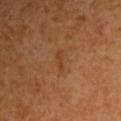Background:
On the right upper arm. A male patient, aged approximately 60. Cropped from a total-body skin-imaging series; the visible field is about 15 mm.